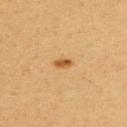Clinical impression:
No biopsy was performed on this lesion — it was imaged during a full skin examination and was not determined to be concerning.